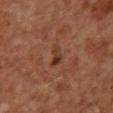Q: Is there a histopathology result?
A: imaged on a skin check; not biopsied
Q: What did automated image analysis measure?
A: an area of roughly 3.5 mm², an outline eccentricity of about 0.85 (0 = round, 1 = elongated), and a shape-asymmetry score of about 0.45 (0 = symmetric); a mean CIELAB color near L≈32 a*≈21 b*≈27, roughly 7 lightness units darker than nearby skin, and a normalized border contrast of about 7; a border-irregularity rating of about 4.5/10, internal color variation of about 2 on a 0–10 scale, and radial color variation of about 0.5; a classifier nevus-likeness of about 0/100 and a lesion-detection confidence of about 100/100
Q: Patient demographics?
A: male, roughly 60 years of age
Q: Illumination type?
A: cross-polarized
Q: How large is the lesion?
A: about 3 mm
Q: Lesion location?
A: the front of the torso
Q: How was this image acquired?
A: 15 mm crop, total-body photography